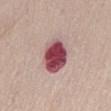Impression:
Imaged during a routine full-body skin examination; the lesion was not biopsied and no histopathology is available.
Context:
The lesion's longest dimension is about 4 mm. A region of skin cropped from a whole-body photographic capture, roughly 15 mm wide. Automated image analysis of the tile measured a footprint of about 12 mm², a shape eccentricity near 0.55, and two-axis asymmetry of about 0.1. The analysis additionally found border irregularity of about 1 on a 0–10 scale, internal color variation of about 6 on a 0–10 scale, and a peripheral color-asymmetry measure near 1.5. On the abdomen. A male patient, aged 73–77. This is a white-light tile.